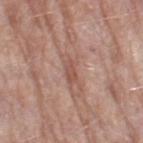{
  "biopsy_status": "not biopsied; imaged during a skin examination",
  "image": {
    "source": "total-body photography crop",
    "field_of_view_mm": 15
  },
  "patient": {
    "sex": "female",
    "age_approx": 70
  },
  "site": "left thigh"
}